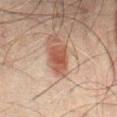Background:
Cropped from a whole-body photographic skin survey; the tile spans about 15 mm. This is a cross-polarized tile. An algorithmic analysis of the crop reported a border-irregularity index near 2/10 and radial color variation of about 0.5. It also reported an automated nevus-likeness rating near 95 out of 100 and a lesion-detection confidence of about 100/100. The patient is a male aged approximately 70. The lesion is on the lower back.